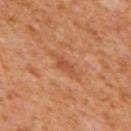follow-up: imaged on a skin check; not biopsied | patient: male, in their mid-60s | acquisition: ~15 mm tile from a whole-body skin photo | diameter: about 2.5 mm | body site: the mid back.